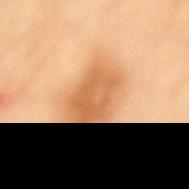This lesion was catalogued during total-body skin photography and was not selected for biopsy. The lesion's longest dimension is about 6 mm. From the mid back. This is a cross-polarized tile. An algorithmic analysis of the crop reported border irregularity of about 3 on a 0–10 scale and a within-lesion color-variation index near 3.5/10. And it measured a detector confidence of about 100 out of 100 that the crop contains a lesion. The subject is a female aged 78 to 82. A 15 mm close-up tile from a total-body photography series done for melanoma screening.